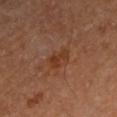This image is a 15 mm lesion crop taken from a total-body photograph. The lesion is located on the left upper arm. The subject is a male aged approximately 65.